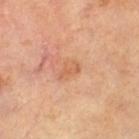Q: Was this lesion biopsied?
A: imaged on a skin check; not biopsied
Q: Illumination type?
A: cross-polarized
Q: What kind of image is this?
A: ~15 mm tile from a whole-body skin photo
Q: What are the patient's age and sex?
A: male, about 65 years old
Q: Lesion size?
A: ~3 mm (longest diameter)